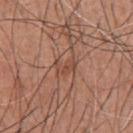The lesion was photographed on a routine skin check and not biopsied; there is no pathology result.
A male subject aged 53 to 57.
The total-body-photography lesion software estimated a footprint of about 4 mm², an outline eccentricity of about 0.75 (0 = round, 1 = elongated), and a symmetry-axis asymmetry near 0.45. And it measured an average lesion color of about L≈48 a*≈22 b*≈29 (CIELAB) and a lesion-to-skin contrast of about 5.5 (normalized; higher = more distinct). The software also gave a color-variation rating of about 1/10 and a peripheral color-asymmetry measure near 0.5.
Located on the chest.
A 15 mm crop from a total-body photograph taken for skin-cancer surveillance.
Captured under white-light illumination.